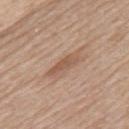follow-up = imaged on a skin check; not biopsied
body site = the upper back
acquisition = 15 mm crop, total-body photography
diameter = ~4.5 mm (longest diameter)
patient = male, roughly 60 years of age
lighting = white-light illumination